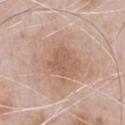Imaged during a routine full-body skin examination; the lesion was not biopsied and no histopathology is available.
On the front of the torso.
A region of skin cropped from a whole-body photographic capture, roughly 15 mm wide.
A male patient aged approximately 50.